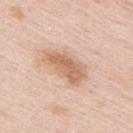A close-up tile cropped from a whole-body skin photograph, about 15 mm across. The patient is a female about 65 years old. The total-body-photography lesion software estimated a footprint of about 12 mm², an outline eccentricity of about 0.85 (0 = round, 1 = elongated), and a shape-asymmetry score of about 0.25 (0 = symmetric). And it measured an average lesion color of about L≈65 a*≈20 b*≈32 (CIELAB), about 11 CIELAB-L* units darker than the surrounding skin, and a normalized border contrast of about 7.5. And it measured a within-lesion color-variation index near 3/10 and peripheral color asymmetry of about 1. It also reported lesion-presence confidence of about 100/100. The lesion's longest dimension is about 5 mm. On the right upper arm.